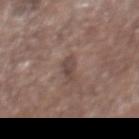Notes:
- workup: catalogued during a skin exam; not biopsied
- image: total-body-photography crop, ~15 mm field of view
- lighting: white-light illumination
- body site: the abdomen
- subject: male, aged 68 to 72